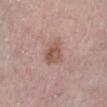size = about 3 mm | TBP lesion metrics = a symmetry-axis asymmetry near 0.3; a classifier nevus-likeness of about 20/100 and lesion-presence confidence of about 100/100 | tile lighting = white-light illumination | patient = female, roughly 65 years of age | acquisition = ~15 mm crop, total-body skin-cancer survey | location = the left lower leg.